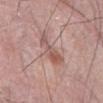site: abdomen
lesion_size:
  long_diameter_mm_approx: 4.5
image:
  source: total-body photography crop
  field_of_view_mm: 15
lighting: white-light
patient:
  sex: male
  age_approx: 60